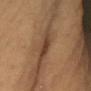{
  "automated_metrics": {
    "border_irregularity_0_10": 4.0,
    "color_variation_0_10": 4.5,
    "peripheral_color_asymmetry": 1.5
  },
  "site": "front of the torso",
  "image": {
    "source": "total-body photography crop",
    "field_of_view_mm": 15
  },
  "lighting": "cross-polarized",
  "patient": {
    "sex": "female",
    "age_approx": 30
  }
}